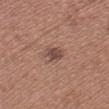This lesion was catalogued during total-body skin photography and was not selected for biopsy.
A region of skin cropped from a whole-body photographic capture, roughly 15 mm wide.
The subject is a male aged around 35.
The lesion is on the head or neck.